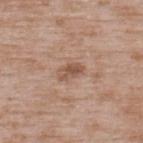| field | value |
|---|---|
| lesion diameter | about 3 mm |
| subject | male, approximately 50 years of age |
| anatomic site | the upper back |
| image-analysis metrics | a lesion color around L≈52 a*≈20 b*≈29 in CIELAB and about 10 CIELAB-L* units darker than the surrounding skin; a nevus-likeness score of about 25/100 and a lesion-detection confidence of about 100/100 |
| lighting | white-light illumination |
| image | ~15 mm tile from a whole-body skin photo |